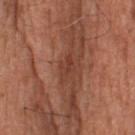Background: A 15 mm crop from a total-body photograph taken for skin-cancer surveillance. The patient is a male about 80 years old. Approximately 3 mm at its widest. The lesion is on the head or neck.